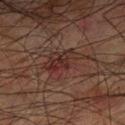Findings:
– follow-up: no biopsy performed (imaged during a skin exam)